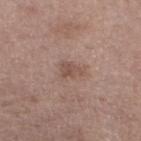biopsy status = no biopsy performed (imaged during a skin exam)
image = 15 mm crop, total-body photography
lesion size = ~3 mm (longest diameter)
tile lighting = white-light illumination
patient = female, roughly 65 years of age
site = the left thigh